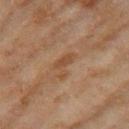Q: Was this lesion biopsied?
A: no biopsy performed (imaged during a skin exam)
Q: Where on the body is the lesion?
A: the right thigh
Q: What kind of image is this?
A: ~15 mm crop, total-body skin-cancer survey
Q: What are the patient's age and sex?
A: female, aged 58 to 62
Q: What did automated image analysis measure?
A: a mean CIELAB color near L≈44 a*≈18 b*≈31 and a normalized border contrast of about 5.5; a border-irregularity rating of about 6.5/10, a within-lesion color-variation index near 1/10, and peripheral color asymmetry of about 0; a lesion-detection confidence of about 100/100
Q: What lighting was used for the tile?
A: cross-polarized
Q: What is the lesion's diameter?
A: ≈3.5 mm